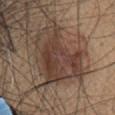Findings:
- notes — catalogued during a skin exam; not biopsied
- acquisition — 15 mm crop, total-body photography
- illumination — white-light illumination
- site — the head or neck
- subject — female, roughly 60 years of age
- diameter — ≈8 mm
- automated lesion analysis — a mean CIELAB color near L≈40 a*≈16 b*≈24, a lesion–skin lightness drop of about 11, and a lesion-to-skin contrast of about 9 (normalized; higher = more distinct); a border-irregularity rating of about 5/10, a within-lesion color-variation index near 6/10, and radial color variation of about 2